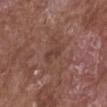{"automated_metrics": {"area_mm2_approx": 2.5, "eccentricity": 0.9, "shape_asymmetry": 0.4, "cielab_L": 39, "cielab_a": 20, "cielab_b": 23, "border_irregularity_0_10": 4.0, "color_variation_0_10": 0.0, "peripheral_color_asymmetry": 0.0}, "site": "right forearm", "image": {"source": "total-body photography crop", "field_of_view_mm": 15}, "lighting": "white-light", "patient": {"sex": "male", "age_approx": 65}, "lesion_size": {"long_diameter_mm_approx": 2.5}}